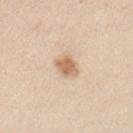The lesion was tiled from a total-body skin photograph and was not biopsied. The subject is a male roughly 45 years of age. Automated image analysis of the tile measured peripheral color asymmetry of about 0.5. The analysis additionally found a nevus-likeness score of about 90/100. On the arm. A lesion tile, about 15 mm wide, cut from a 3D total-body photograph. This is a white-light tile.